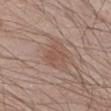  biopsy_status: not biopsied; imaged during a skin examination
  automated_metrics:
    area_mm2_approx: 4.0
    eccentricity: 0.7
    shape_asymmetry: 0.5
    nevus_likeness_0_100: 10
  lesion_size:
    long_diameter_mm_approx: 3.0
  patient:
    sex: male
    age_approx: 35
  site: leg
  image:
    source: total-body photography crop
    field_of_view_mm: 15